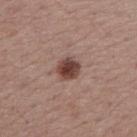Impression: The lesion was photographed on a routine skin check and not biopsied; there is no pathology result. Context: A region of skin cropped from a whole-body photographic capture, roughly 15 mm wide. Longest diameter approximately 3 mm. From the arm. The patient is a female aged around 55. The total-body-photography lesion software estimated a lesion area of about 6 mm². It also reported an automated nevus-likeness rating near 95 out of 100 and a detector confidence of about 100 out of 100 that the crop contains a lesion.